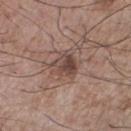Assessment:
Recorded during total-body skin imaging; not selected for excision or biopsy.
Acquisition and patient details:
On the front of the torso. A 15 mm close-up extracted from a 3D total-body photography capture. A male subject in their mid- to late 70s. The recorded lesion diameter is about 3 mm. The tile uses white-light illumination.